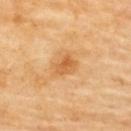• subject: female, aged around 60
• image: total-body-photography crop, ~15 mm field of view
• anatomic site: the upper back